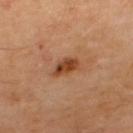follow-up: imaged on a skin check; not biopsied | body site: the upper back | TBP lesion metrics: an eccentricity of roughly 0.8 and two-axis asymmetry of about 0.3; a border-irregularity index near 2.5/10 and peripheral color asymmetry of about 1.5; a classifier nevus-likeness of about 85/100 and a detector confidence of about 100 out of 100 that the crop contains a lesion | image source: total-body-photography crop, ~15 mm field of view | lesion size: ~3 mm (longest diameter) | illumination: cross-polarized illumination | patient: male, aged around 65.